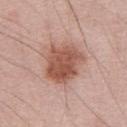The lesion was tiled from a total-body skin photograph and was not biopsied. This image is a 15 mm lesion crop taken from a total-body photograph. The lesion's longest dimension is about 5 mm. Automated image analysis of the tile measured a footprint of about 18 mm², an outline eccentricity of about 0.5 (0 = round, 1 = elongated), and two-axis asymmetry of about 0.2. It also reported a within-lesion color-variation index near 4.5/10 and radial color variation of about 1.5. The tile uses white-light illumination. Located on the front of the torso. The subject is a male aged approximately 55.